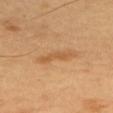Q: Was a biopsy performed?
A: imaged on a skin check; not biopsied
Q: What are the patient's age and sex?
A: male, aged 48–52
Q: Where on the body is the lesion?
A: the upper back
Q: What did automated image analysis measure?
A: an eccentricity of roughly 0.95 and a shape-asymmetry score of about 0.3 (0 = symmetric); a lesion color around L≈56 a*≈21 b*≈40 in CIELAB and a normalized border contrast of about 5.5; lesion-presence confidence of about 100/100
Q: Illumination type?
A: cross-polarized illumination
Q: How was this image acquired?
A: 15 mm crop, total-body photography
Q: How large is the lesion?
A: ≈4 mm